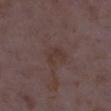This lesion was catalogued during total-body skin photography and was not selected for biopsy. The patient is a female in their mid-30s. Located on the right lower leg. Cropped from a whole-body photographic skin survey; the tile spans about 15 mm. The recorded lesion diameter is about 2.5 mm.